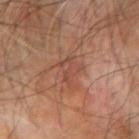{
  "biopsy_status": "not biopsied; imaged during a skin examination",
  "image": {
    "source": "total-body photography crop",
    "field_of_view_mm": 15
  },
  "patient": {
    "sex": "male",
    "age_approx": 70
  },
  "lighting": "cross-polarized",
  "site": "left forearm"
}